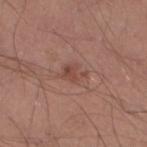Impression:
No biopsy was performed on this lesion — it was imaged during a full skin examination and was not determined to be concerning.
Acquisition and patient details:
The subject is a male approximately 30 years of age. A region of skin cropped from a whole-body photographic capture, roughly 15 mm wide. Imaged with white-light lighting. The lesion is on the left lower leg.